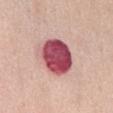{"biopsy_status": "not biopsied; imaged during a skin examination", "lighting": "white-light", "site": "chest", "automated_metrics": {"cielab_L": 50, "cielab_a": 35, "cielab_b": 19, "vs_skin_contrast_norm": 14.5}, "patient": {"sex": "female", "age_approx": 60}, "image": {"source": "total-body photography crop", "field_of_view_mm": 15}}